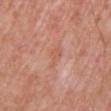Captured during whole-body skin photography for melanoma surveillance; the lesion was not biopsied. Imaged with white-light lighting. A roughly 15 mm field-of-view crop from a total-body skin photograph. The recorded lesion diameter is about 2.5 mm. A male subject roughly 85 years of age or older. Located on the chest.